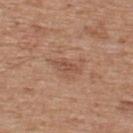Notes:
– notes — no biopsy performed (imaged during a skin exam)